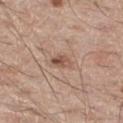Assessment: Part of a total-body skin-imaging series; this lesion was reviewed on a skin check and was not flagged for biopsy. Background: A close-up tile cropped from a whole-body skin photograph, about 15 mm across. Approximately 2.5 mm at its widest. Captured under white-light illumination. A male patient, aged around 60. Automated image analysis of the tile measured a footprint of about 3 mm². The analysis additionally found a lesion color around L≈52 a*≈20 b*≈28 in CIELAB, roughly 11 lightness units darker than nearby skin, and a normalized lesion–skin contrast near 7.5. The software also gave a within-lesion color-variation index near 4.5/10 and radial color variation of about 2. And it measured an automated nevus-likeness rating near 45 out of 100 and a detector confidence of about 100 out of 100 that the crop contains a lesion. On the right thigh.